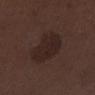biopsy status = no biopsy performed (imaged during a skin exam)
tile lighting = white-light illumination
diameter = about 5 mm
subject = male, about 70 years old
image = total-body-photography crop, ~15 mm field of view
body site = the right lower leg